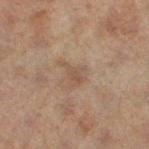Findings:
- patient · female, aged 58 to 62
- anatomic site · the left thigh
- imaging modality · 15 mm crop, total-body photography
- TBP lesion metrics · a footprint of about 4 mm² and two-axis asymmetry of about 0.55; a lesion color around L≈45 a*≈14 b*≈26 in CIELAB, a lesion–skin lightness drop of about 6, and a lesion-to-skin contrast of about 5 (normalized; higher = more distinct); a classifier nevus-likeness of about 0/100 and lesion-presence confidence of about 100/100
- illumination · cross-polarized illumination